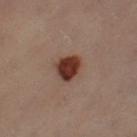Q: Was this lesion biopsied?
A: imaged on a skin check; not biopsied
Q: How was the tile lit?
A: cross-polarized illumination
Q: What are the patient's age and sex?
A: female, roughly 50 years of age
Q: What is the imaging modality?
A: ~15 mm crop, total-body skin-cancer survey
Q: Lesion location?
A: the right lower leg
Q: Lesion size?
A: ~3 mm (longest diameter)